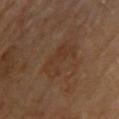Findings:
– follow-up · total-body-photography surveillance lesion; no biopsy
– image-analysis metrics · an area of roughly 9.5 mm²; an average lesion color of about L≈36 a*≈18 b*≈29 (CIELAB), about 5 CIELAB-L* units darker than the surrounding skin, and a lesion-to-skin contrast of about 5 (normalized; higher = more distinct); lesion-presence confidence of about 100/100
– subject · female, aged approximately 70
– body site · the upper back
– tile lighting · cross-polarized
– imaging modality · ~15 mm tile from a whole-body skin photo